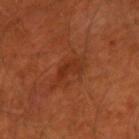Clinical impression:
No biopsy was performed on this lesion — it was imaged during a full skin examination and was not determined to be concerning.
Image and clinical context:
A lesion tile, about 15 mm wide, cut from a 3D total-body photograph. The tile uses cross-polarized illumination. The lesion is located on the left arm. An algorithmic analysis of the crop reported an average lesion color of about L≈32 a*≈29 b*≈35 (CIELAB), a lesion–skin lightness drop of about 6, and a normalized lesion–skin contrast near 6. The software also gave border irregularity of about 4 on a 0–10 scale, a within-lesion color-variation index near 1.5/10, and peripheral color asymmetry of about 0.5. And it measured a nevus-likeness score of about 0/100. The patient is a male aged 48 to 52.